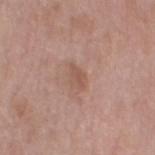<tbp_lesion>
<biopsy_status>not biopsied; imaged during a skin examination</biopsy_status>
<automated_metrics>
  <eccentricity>0.75</eccentricity>
  <shape_asymmetry>0.25</shape_asymmetry>
  <cielab_L>54</cielab_L>
  <cielab_a>20</cielab_a>
  <cielab_b>27</cielab_b>
  <vs_skin_darker_L>7.0</vs_skin_darker_L>
  <vs_skin_contrast_norm>5.5</vs_skin_contrast_norm>
  <border_irregularity_0_10>2.5</border_irregularity_0_10>
  <color_variation_0_10>1.5</color_variation_0_10>
  <peripheral_color_asymmetry>0.5</peripheral_color_asymmetry>
</automated_metrics>
<site>left thigh</site>
<lesion_size>
  <long_diameter_mm_approx>2.5</long_diameter_mm_approx>
</lesion_size>
<image>
  <source>total-body photography crop</source>
  <field_of_view_mm>15</field_of_view_mm>
</image>
<lighting>white-light</lighting>
<patient>
  <sex>female</sex>
  <age_approx>70</age_approx>
</patient>
</tbp_lesion>